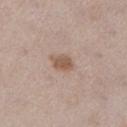{
  "biopsy_status": "not biopsied; imaged during a skin examination"
}